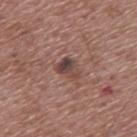This is a white-light tile.
Located on the upper back.
A male subject aged around 55.
The total-body-photography lesion software estimated an automated nevus-likeness rating near 65 out of 100 and lesion-presence confidence of about 100/100.
A roughly 15 mm field-of-view crop from a total-body skin photograph.
Approximately 3 mm at its widest.